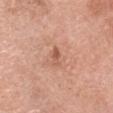The lesion was photographed on a routine skin check and not biopsied; there is no pathology result. A roughly 15 mm field-of-view crop from a total-body skin photograph. From the head or neck. The subject is a female aged around 60.